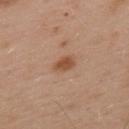<record>
<biopsy_status>not biopsied; imaged during a skin examination</biopsy_status>
<site>upper back</site>
<image>
  <source>total-body photography crop</source>
  <field_of_view_mm>15</field_of_view_mm>
</image>
<lesion_size>
  <long_diameter_mm_approx>2.5</long_diameter_mm_approx>
</lesion_size>
<automated_metrics>
  <vs_skin_darker_L>10.0</vs_skin_darker_L>
  <vs_skin_contrast_norm>8.5</vs_skin_contrast_norm>
  <lesion_detection_confidence_0_100>100</lesion_detection_confidence_0_100>
</automated_metrics>
<patient>
  <sex>male</sex>
  <age_approx>55</age_approx>
</patient>
<lighting>white-light</lighting>
</record>